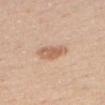Assessment:
The lesion was tiled from a total-body skin photograph and was not biopsied.
Clinical summary:
Approximately 3.5 mm at its widest. Imaged with white-light lighting. A region of skin cropped from a whole-body photographic capture, roughly 15 mm wide. The subject is a male approximately 30 years of age. Located on the chest.